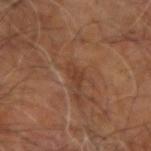Imaged during a routine full-body skin examination; the lesion was not biopsied and no histopathology is available.
This is a cross-polarized tile.
A 15 mm close-up tile from a total-body photography series done for melanoma screening.
About 3 mm across.
An algorithmic analysis of the crop reported an outline eccentricity of about 0.8 (0 = round, 1 = elongated) and a shape-asymmetry score of about 0.5 (0 = symmetric). It also reported a classifier nevus-likeness of about 0/100 and lesion-presence confidence of about 90/100.
The lesion is on the right arm.
A male patient, aged 58–62.